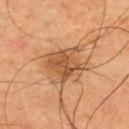– workup · no biopsy performed (imaged during a skin exam)
– location · the upper back
– imaging modality · ~15 mm tile from a whole-body skin photo
– patient · male, aged around 50